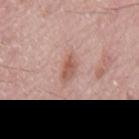workup: imaged on a skin check; not biopsied | body site: the back | subject: male, about 75 years old | automated lesion analysis: a nevus-likeness score of about 30/100 and a detector confidence of about 100 out of 100 that the crop contains a lesion | illumination: white-light | image source: ~15 mm crop, total-body skin-cancer survey.